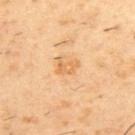Findings:
* notes: no biopsy performed (imaged during a skin exam)
* body site: the upper back
* illumination: cross-polarized illumination
* size: about 2.5 mm
* patient: male, about 55 years old
* image: total-body-photography crop, ~15 mm field of view